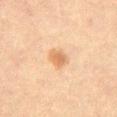Assessment:
The lesion was tiled from a total-body skin photograph and was not biopsied.